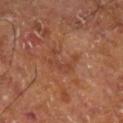{
  "biopsy_status": "not biopsied; imaged during a skin examination",
  "patient": {
    "sex": "male",
    "age_approx": 60
  },
  "site": "right leg",
  "automated_metrics": {
    "eccentricity": 0.65,
    "cielab_L": 41,
    "cielab_a": 24,
    "cielab_b": 30,
    "border_irregularity_0_10": 9.5,
    "color_variation_0_10": 0.0,
    "peripheral_color_asymmetry": 0.0
  },
  "lesion_size": {
    "long_diameter_mm_approx": 3.5
  },
  "image": {
    "source": "total-body photography crop",
    "field_of_view_mm": 15
  }
}